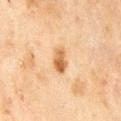Impression:
Recorded during total-body skin imaging; not selected for excision or biopsy.
Image and clinical context:
Longest diameter approximately 3 mm. A region of skin cropped from a whole-body photographic capture, roughly 15 mm wide. This is a cross-polarized tile. A male subject, aged 43 to 47. The lesion is on the mid back.